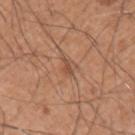Case summary:
• automated lesion analysis · an eccentricity of roughly 0.95 and a symmetry-axis asymmetry near 0.45; an average lesion color of about L≈50 a*≈22 b*≈31 (CIELAB), roughly 8 lightness units darker than nearby skin, and a normalized border contrast of about 6; border irregularity of about 4.5 on a 0–10 scale, a color-variation rating of about 0/10, and peripheral color asymmetry of about 0
• diameter · about 2.5 mm
• acquisition · ~15 mm tile from a whole-body skin photo
• subject · male, roughly 60 years of age
• anatomic site · the left upper arm